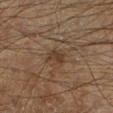The patient is a male in their mid-60s.
Cropped from a total-body skin-imaging series; the visible field is about 15 mm.
Longest diameter approximately 2.5 mm.
An algorithmic analysis of the crop reported a nevus-likeness score of about 5/100 and a detector confidence of about 95 out of 100 that the crop contains a lesion.
Located on the leg.